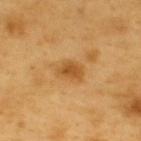Assessment:
Recorded during total-body skin imaging; not selected for excision or biopsy.
Clinical summary:
The lesion's longest dimension is about 3 mm. A 15 mm close-up tile from a total-body photography series done for melanoma screening. A male subject, roughly 60 years of age. The lesion-visualizer software estimated a lesion color around L≈52 a*≈22 b*≈45 in CIELAB and a normalized border contrast of about 7.5. The analysis additionally found a border-irregularity index near 2.5/10, a within-lesion color-variation index near 2.5/10, and peripheral color asymmetry of about 1. The analysis additionally found a lesion-detection confidence of about 100/100. This is a cross-polarized tile. The lesion is located on the back.